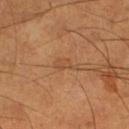workup: total-body-photography surveillance lesion; no biopsy | location: the left lower leg | acquisition: ~15 mm crop, total-body skin-cancer survey | lesion size: ≈3 mm | illumination: cross-polarized | patient: male, aged around 55.